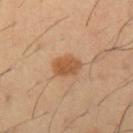The lesion was tiled from a total-body skin photograph and was not biopsied. Automated tile analysis of the lesion measured a lesion color around L≈52 a*≈21 b*≈36 in CIELAB, roughly 10 lightness units darker than nearby skin, and a lesion-to-skin contrast of about 8 (normalized; higher = more distinct). And it measured a border-irregularity index near 2/10 and a within-lesion color-variation index near 2/10. The patient is a male about 40 years old. The lesion is located on the left upper arm. Measured at roughly 3.5 mm in maximum diameter. A lesion tile, about 15 mm wide, cut from a 3D total-body photograph.